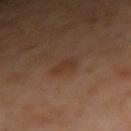This lesion was catalogued during total-body skin photography and was not selected for biopsy. The patient is a female in their 40s. A roughly 15 mm field-of-view crop from a total-body skin photograph. On the right forearm. Automated tile analysis of the lesion measured an area of roughly 4 mm², an eccentricity of roughly 0.65, and a shape-asymmetry score of about 0.3 (0 = symmetric). It also reported an average lesion color of about L≈34 a*≈18 b*≈27 (CIELAB), roughly 5 lightness units darker than nearby skin, and a normalized lesion–skin contrast near 5. And it measured a classifier nevus-likeness of about 15/100 and lesion-presence confidence of about 100/100. Captured under cross-polarized illumination.